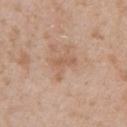workup: catalogued during a skin exam; not biopsied | site: the right upper arm | patient: male, aged around 50 | lighting: white-light | acquisition: 15 mm crop, total-body photography.